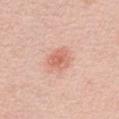Assessment: Imaged during a routine full-body skin examination; the lesion was not biopsied and no histopathology is available. Context: Cropped from a total-body skin-imaging series; the visible field is about 15 mm. This is a white-light tile. About 3 mm across. A male patient in their mid-60s. The lesion-visualizer software estimated border irregularity of about 2.5 on a 0–10 scale, a color-variation rating of about 3/10, and a peripheral color-asymmetry measure near 1. From the chest.